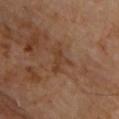Impression: The lesion was tiled from a total-body skin photograph and was not biopsied. Clinical summary: The subject is a male about 60 years old. This image is a 15 mm lesion crop taken from a total-body photograph. Automated tile analysis of the lesion measured an area of roughly 4.5 mm², an outline eccentricity of about 0.65 (0 = round, 1 = elongated), and two-axis asymmetry of about 0.55. The analysis additionally found a lesion color around L≈37 a*≈19 b*≈29 in CIELAB, a lesion–skin lightness drop of about 6, and a normalized lesion–skin contrast near 6. It also reported a border-irregularity rating of about 6.5/10, a within-lesion color-variation index near 0.5/10, and peripheral color asymmetry of about 0. The analysis additionally found a nevus-likeness score of about 0/100 and lesion-presence confidence of about 100/100. The lesion is on the chest. The tile uses cross-polarized illumination.